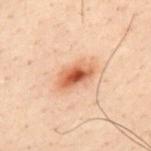Case summary:
• illumination — cross-polarized
• site — the mid back
• lesion size — ≈4 mm
• patient — male, aged 28 to 32
• acquisition — ~15 mm tile from a whole-body skin photo
• automated metrics — a border-irregularity rating of about 2/10, internal color variation of about 7.5 on a 0–10 scale, and a peripheral color-asymmetry measure near 2